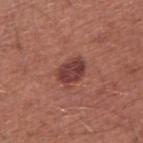Clinical impression:
The lesion was tiled from a total-body skin photograph and was not biopsied.
Image and clinical context:
The lesion is on the right upper arm. A male patient, aged 58–62. A 15 mm crop from a total-body photograph taken for skin-cancer surveillance. The tile uses white-light illumination. The lesion-visualizer software estimated two-axis asymmetry of about 0.25. And it measured a lesion color around L≈40 a*≈25 b*≈24 in CIELAB, about 12 CIELAB-L* units darker than the surrounding skin, and a normalized border contrast of about 9.5. It also reported a border-irregularity rating of about 2.5/10, internal color variation of about 4 on a 0–10 scale, and radial color variation of about 1.5. It also reported lesion-presence confidence of about 100/100.